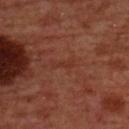Located on the chest.
A male patient aged 58 to 62.
A roughly 15 mm field-of-view crop from a total-body skin photograph.